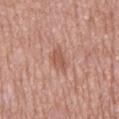The lesion was photographed on a routine skin check and not biopsied; there is no pathology result. The lesion-visualizer software estimated an eccentricity of roughly 0.85 and a symmetry-axis asymmetry near 0.25. It also reported a lesion color around L≈55 a*≈23 b*≈28 in CIELAB, roughly 8 lightness units darker than nearby skin, and a lesion-to-skin contrast of about 6 (normalized; higher = more distinct). It also reported a border-irregularity index near 3/10. The analysis additionally found an automated nevus-likeness rating near 5 out of 100 and a detector confidence of about 100 out of 100 that the crop contains a lesion. The lesion is on the mid back. About 3.5 mm across. A male patient, aged 63–67. A 15 mm crop from a total-body photograph taken for skin-cancer surveillance. The tile uses white-light illumination.